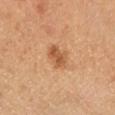<lesion>
<biopsy_status>not biopsied; imaged during a skin examination</biopsy_status>
</lesion>